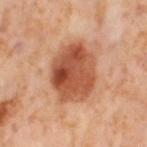Assessment: The lesion was tiled from a total-body skin photograph and was not biopsied. Clinical summary: From the right thigh. A female patient, aged approximately 55. Longest diameter approximately 6.5 mm. This is a cross-polarized tile. A close-up tile cropped from a whole-body skin photograph, about 15 mm across. Automated tile analysis of the lesion measured internal color variation of about 8.5 on a 0–10 scale.